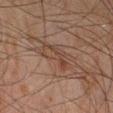{
  "biopsy_status": "not biopsied; imaged during a skin examination",
  "image": {
    "source": "total-body photography crop",
    "field_of_view_mm": 15
  },
  "lesion_size": {
    "long_diameter_mm_approx": 6.5
  },
  "patient": {
    "sex": "male",
    "age_approx": 45
  },
  "automated_metrics": {
    "area_mm2_approx": 11.0,
    "eccentricity": 0.9,
    "shape_asymmetry": 0.35,
    "border_irregularity_0_10": 5.0,
    "color_variation_0_10": 4.5,
    "peripheral_color_asymmetry": 1.5
  },
  "site": "right lower leg",
  "lighting": "cross-polarized"
}